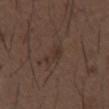biopsy status=total-body-photography surveillance lesion; no biopsy | anatomic site=the abdomen | subject=male, aged around 50 | image source=15 mm crop, total-body photography | diameter=about 3 mm.